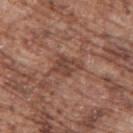{
  "biopsy_status": "not biopsied; imaged during a skin examination",
  "image": {
    "source": "total-body photography crop",
    "field_of_view_mm": 15
  },
  "site": "upper back",
  "lighting": "white-light",
  "patient": {
    "sex": "male",
    "age_approx": 75
  }
}